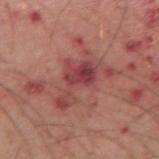Assessment:
Imaged during a routine full-body skin examination; the lesion was not biopsied and no histopathology is available.
Context:
The lesion is located on the right upper arm. An algorithmic analysis of the crop reported a lesion area of about 13 mm² and a shape-asymmetry score of about 0.65 (0 = symmetric). The lesion's longest dimension is about 7 mm. Cropped from a whole-body photographic skin survey; the tile spans about 15 mm. A male subject in their 50s.